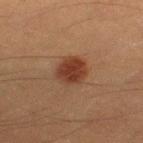The lesion was tiled from a total-body skin photograph and was not biopsied.
A male subject roughly 40 years of age.
Cropped from a total-body skin-imaging series; the visible field is about 15 mm.
On the right thigh.